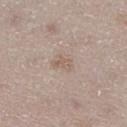No biopsy was performed on this lesion — it was imaged during a full skin examination and was not determined to be concerning. On the right lower leg. A female subject, roughly 50 years of age. Cropped from a whole-body photographic skin survey; the tile spans about 15 mm.